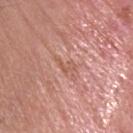follow-up: no biopsy performed (imaged during a skin exam) | automated lesion analysis: an area of roughly 2.5 mm², an outline eccentricity of about 0.8 (0 = round, 1 = elongated), and two-axis asymmetry of about 0.5; a border-irregularity index near 5/10, a color-variation rating of about 0/10, and peripheral color asymmetry of about 0; a nevus-likeness score of about 0/100 and a lesion-detection confidence of about 65/100 | lesion size: ≈2.5 mm | imaging modality: ~15 mm tile from a whole-body skin photo | patient: female, aged around 60 | tile lighting: white-light | location: the head or neck.